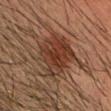The lesion was photographed on a routine skin check and not biopsied; there is no pathology result. Automated image analysis of the tile measured an eccentricity of roughly 0.7. The analysis additionally found a classifier nevus-likeness of about 100/100 and a detector confidence of about 100 out of 100 that the crop contains a lesion. A male subject roughly 40 years of age. Cropped from a total-body skin-imaging series; the visible field is about 15 mm. From the head or neck. This is a cross-polarized tile.